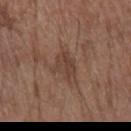patient = female, aged 78–82 | tile lighting = white-light | location = the left forearm | acquisition = total-body-photography crop, ~15 mm field of view.